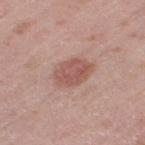Case summary:
* follow-up · no biopsy performed (imaged during a skin exam)
* lesion size · ≈4.5 mm
* image source · ~15 mm crop, total-body skin-cancer survey
* subject · female, aged around 40
* automated lesion analysis · an area of roughly 9.5 mm², an eccentricity of roughly 0.75, and two-axis asymmetry of about 0.1; about 10 CIELAB-L* units darker than the surrounding skin and a normalized border contrast of about 7; border irregularity of about 1.5 on a 0–10 scale, a within-lesion color-variation index near 3/10, and a peripheral color-asymmetry measure near 1; lesion-presence confidence of about 100/100
* site · the leg
* lighting · white-light illumination